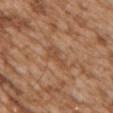No biopsy was performed on this lesion — it was imaged during a full skin examination and was not determined to be concerning. Captured under white-light illumination. The lesion is located on the chest. Approximately 3.5 mm at its widest. A 15 mm crop from a total-body photograph taken for skin-cancer surveillance. A female subject approximately 30 years of age.